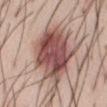A 15 mm close-up extracted from a 3D total-body photography capture.
A male patient in their mid-30s.
The lesion is located on the front of the torso.
Captured under white-light illumination.